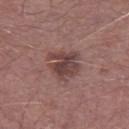{"biopsy_status": "not biopsied; imaged during a skin examination", "image": {"source": "total-body photography crop", "field_of_view_mm": 15}, "lesion_size": {"long_diameter_mm_approx": 4.0}, "patient": {"sex": "male", "age_approx": 70}, "lighting": "white-light", "site": "right lower leg", "automated_metrics": {"vs_skin_darker_L": 10.0, "vs_skin_contrast_norm": 8.0, "nevus_likeness_0_100": 35, "lesion_detection_confidence_0_100": 100}}